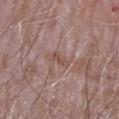Case summary:
– workup · total-body-photography surveillance lesion; no biopsy
– acquisition · 15 mm crop, total-body photography
– patient · male, about 65 years old
– site · the arm
– illumination · white-light
– automated metrics · an area of roughly 3 mm², an outline eccentricity of about 0.85 (0 = round, 1 = elongated), and two-axis asymmetry of about 0.3; a nevus-likeness score of about 0/100 and a detector confidence of about 100 out of 100 that the crop contains a lesion